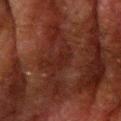follow-up — imaged on a skin check; not biopsied | anatomic site — the right upper arm | acquisition — total-body-photography crop, ~15 mm field of view | subject — male, aged 78–82.